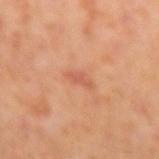{"biopsy_status": "not biopsied; imaged during a skin examination", "lighting": "cross-polarized", "site": "right forearm", "patient": {"sex": "female", "age_approx": 50}, "image": {"source": "total-body photography crop", "field_of_view_mm": 15}, "automated_metrics": {"area_mm2_approx": 2.0, "eccentricity": 0.9, "shape_asymmetry": 0.3, "cielab_L": 56, "cielab_a": 28, "cielab_b": 33, "vs_skin_darker_L": 8.0, "nevus_likeness_0_100": 0, "lesion_detection_confidence_0_100": 100}, "lesion_size": {"long_diameter_mm_approx": 2.5}}